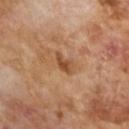  biopsy_status: not biopsied; imaged during a skin examination
  lighting: cross-polarized
  image:
    source: total-body photography crop
    field_of_view_mm: 15
  automated_metrics:
    area_mm2_approx: 3.5
    eccentricity: 0.8
    shape_asymmetry: 0.35
    nevus_likeness_0_100: 25
    lesion_detection_confidence_0_100: 100
  lesion_size:
    long_diameter_mm_approx: 2.5
  patient:
    sex: male
    age_approx: 65